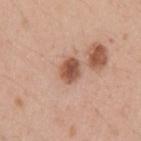Recorded during total-body skin imaging; not selected for excision or biopsy. The lesion's longest dimension is about 2.5 mm. The lesion is on the arm. The tile uses white-light illumination. Cropped from a total-body skin-imaging series; the visible field is about 15 mm. A male patient, aged 28 to 32. The total-body-photography lesion software estimated a shape eccentricity near 0.45 and a shape-asymmetry score of about 0.1 (0 = symmetric). It also reported an average lesion color of about L≈53 a*≈22 b*≈30 (CIELAB), about 14 CIELAB-L* units darker than the surrounding skin, and a normalized border contrast of about 9.5.